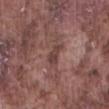Impression:
This lesion was catalogued during total-body skin photography and was not selected for biopsy.
Image and clinical context:
Imaged with white-light lighting. From the front of the torso. A close-up tile cropped from a whole-body skin photograph, about 15 mm across. A male patient, about 75 years old.